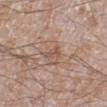Part of a total-body skin-imaging series; this lesion was reviewed on a skin check and was not flagged for biopsy.
On the left lower leg.
A male subject aged around 60.
A 15 mm close-up tile from a total-body photography series done for melanoma screening.